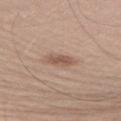| feature | finding |
|---|---|
| biopsy status | imaged on a skin check; not biopsied |
| image source | 15 mm crop, total-body photography |
| anatomic site | the right upper arm |
| subject | male, aged around 40 |